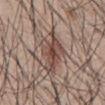lighting: white-light
image:
  source: total-body photography crop
  field_of_view_mm: 15
lesion_size:
  long_diameter_mm_approx: 6.5
site: mid back
patient:
  sex: male
  age_approx: 45